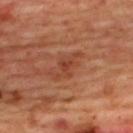follow-up: no biopsy performed (imaged during a skin exam); site: the upper back; illumination: cross-polarized; diameter: ~4 mm (longest diameter); image: ~15 mm tile from a whole-body skin photo; TBP lesion metrics: a normalized lesion–skin contrast near 5.5; patient: female, in their mid-40s.